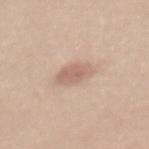| key | value |
|---|---|
| workup | total-body-photography surveillance lesion; no biopsy |
| tile lighting | white-light illumination |
| lesion size | ~3.5 mm (longest diameter) |
| body site | the upper back |
| subject | female, about 35 years old |
| image | ~15 mm crop, total-body skin-cancer survey |